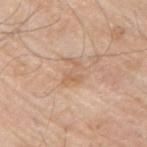The lesion was photographed on a routine skin check and not biopsied; there is no pathology result.
This is a white-light tile.
A male subject, about 65 years old.
Located on the arm.
This image is a 15 mm lesion crop taken from a total-body photograph.